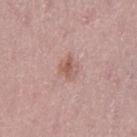Q: Was a biopsy performed?
A: catalogued during a skin exam; not biopsied
Q: Illumination type?
A: white-light illumination
Q: What is the anatomic site?
A: the left thigh
Q: How was this image acquired?
A: ~15 mm tile from a whole-body skin photo
Q: Lesion size?
A: ~2.5 mm (longest diameter)
Q: Patient demographics?
A: female, about 50 years old